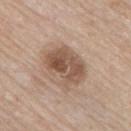Background: From the right upper arm. A 15 mm close-up tile from a total-body photography series done for melanoma screening. The lesion's longest dimension is about 5 mm. A male patient aged 83 or older.